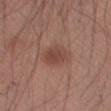Clinical impression: Imaged during a routine full-body skin examination; the lesion was not biopsied and no histopathology is available. Image and clinical context: Automated image analysis of the tile measured a classifier nevus-likeness of about 75/100. Captured under white-light illumination. A male patient, aged 43 to 47. A lesion tile, about 15 mm wide, cut from a 3D total-body photograph. The lesion is on the left upper arm.